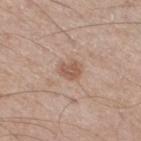Q: Is there a histopathology result?
A: no biopsy performed (imaged during a skin exam)
Q: What are the patient's age and sex?
A: male, roughly 65 years of age
Q: What kind of image is this?
A: ~15 mm crop, total-body skin-cancer survey
Q: Lesion size?
A: ≈2.5 mm
Q: Automated lesion metrics?
A: a footprint of about 4 mm², an outline eccentricity of about 0.65 (0 = round, 1 = elongated), and two-axis asymmetry of about 0.25; a border-irregularity rating of about 2.5/10, a color-variation rating of about 2/10, and a peripheral color-asymmetry measure near 1
Q: Lesion location?
A: the right thigh